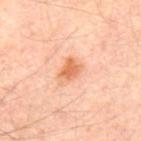• biopsy status: no biopsy performed (imaged during a skin exam)
• size: about 3 mm
• acquisition: ~15 mm tile from a whole-body skin photo
• location: the mid back
• subject: about 55 years old
• lighting: cross-polarized illumination